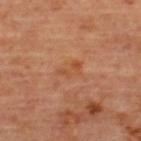Findings:
- follow-up: catalogued during a skin exam; not biopsied
- location: the upper back
- illumination: cross-polarized illumination
- automated lesion analysis: a classifier nevus-likeness of about 0/100 and a detector confidence of about 100 out of 100 that the crop contains a lesion
- lesion size: about 3 mm
- imaging modality: 15 mm crop, total-body photography
- subject: female, approximately 45 years of age